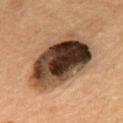Captured under cross-polarized illumination. Automated image analysis of the tile measured border irregularity of about 2.5 on a 0–10 scale, internal color variation of about 10 on a 0–10 scale, and peripheral color asymmetry of about 5.5. A region of skin cropped from a whole-body photographic capture, roughly 15 mm wide. The lesion is on the mid back. The lesion's longest dimension is about 9 mm. A male subject approximately 55 years of age.